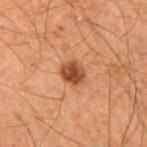workup: no biopsy performed (imaged during a skin exam) | anatomic site: the right upper arm | TBP lesion metrics: a footprint of about 5.5 mm²; border irregularity of about 1 on a 0–10 scale and radial color variation of about 1; lesion-presence confidence of about 100/100 | lighting: cross-polarized illumination | patient: male, aged 63–67 | imaging modality: 15 mm crop, total-body photography | size: ~3 mm (longest diameter).